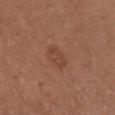The lesion was photographed on a routine skin check and not biopsied; there is no pathology result. The lesion is located on the chest. A female subject, aged 38–42. A close-up tile cropped from a whole-body skin photograph, about 15 mm across.